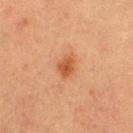<record>
<biopsy_status>not biopsied; imaged during a skin examination</biopsy_status>
<image>
  <source>total-body photography crop</source>
  <field_of_view_mm>15</field_of_view_mm>
</image>
<site>arm</site>
<patient>
  <sex>female</sex>
  <age_approx>55</age_approx>
</patient>
<lesion_size>
  <long_diameter_mm_approx>3.0</long_diameter_mm_approx>
</lesion_size>
<lighting>cross-polarized</lighting>
</record>